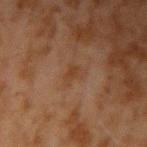About 3 mm across. This is a cross-polarized tile. A region of skin cropped from a whole-body photographic capture, roughly 15 mm wide. On the right upper arm. A male subject aged 43 to 47.